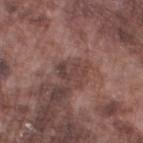Notes:
- biopsy status · no biopsy performed (imaged during a skin exam)
- body site · the left thigh
- diameter · ≈3.5 mm
- tile lighting · white-light
- subject · male, aged 73 to 77
- image-analysis metrics · a footprint of about 4.5 mm², an eccentricity of roughly 0.85, and a symmetry-axis asymmetry near 0.4; a mean CIELAB color near L≈41 a*≈19 b*≈20, a lesion–skin lightness drop of about 8, and a normalized border contrast of about 7; a nevus-likeness score of about 0/100 and lesion-presence confidence of about 85/100
- acquisition · 15 mm crop, total-body photography